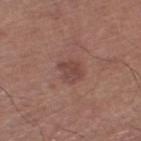Notes:
– tile lighting — white-light
– image-analysis metrics — an area of roughly 4.5 mm², a shape eccentricity near 0.55, and a symmetry-axis asymmetry near 0.3; a mean CIELAB color near L≈44 a*≈21 b*≈23 and roughly 8 lightness units darker than nearby skin
– imaging modality — ~15 mm crop, total-body skin-cancer survey
– lesion diameter — about 2.5 mm
– subject — male, in their 70s
– site — the left thigh